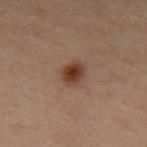This lesion was catalogued during total-body skin photography and was not selected for biopsy.
A male patient, aged approximately 60.
The lesion is on the mid back.
A 15 mm crop from a total-body photograph taken for skin-cancer surveillance.